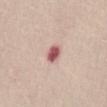Findings:
* workup: imaged on a skin check; not biopsied
* lighting: white-light
* image-analysis metrics: a footprint of about 4 mm² and a shape eccentricity near 0.75; a lesion–skin lightness drop of about 17 and a normalized border contrast of about 10.5; a border-irregularity index near 1.5/10, internal color variation of about 3.5 on a 0–10 scale, and a peripheral color-asymmetry measure near 1; a nevus-likeness score of about 0/100 and lesion-presence confidence of about 100/100
* patient: male, approximately 65 years of age
* body site: the abdomen
* image source: ~15 mm crop, total-body skin-cancer survey
* lesion size: about 3 mm